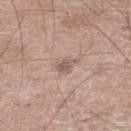  lesion_size:
    long_diameter_mm_approx: 2.5
  lighting: white-light
  image:
    source: total-body photography crop
    field_of_view_mm: 15
  patient:
    sex: male
    age_approx: 60
  site: left lower leg